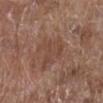Q: Was a biopsy performed?
A: total-body-photography surveillance lesion; no biopsy
Q: What kind of image is this?
A: ~15 mm tile from a whole-body skin photo
Q: Who is the patient?
A: female, roughly 80 years of age
Q: How was the tile lit?
A: white-light
Q: What is the anatomic site?
A: the right lower leg
Q: What is the lesion's diameter?
A: ~4 mm (longest diameter)